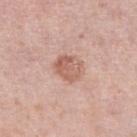Recorded during total-body skin imaging; not selected for excision or biopsy. Captured under white-light illumination. Located on the right lower leg. A female patient in their 50s. This image is a 15 mm lesion crop taken from a total-body photograph. Automated image analysis of the tile measured a mean CIELAB color near L≈60 a*≈22 b*≈27 and a lesion-to-skin contrast of about 6.5 (normalized; higher = more distinct). And it measured a classifier nevus-likeness of about 25/100 and a lesion-detection confidence of about 100/100.